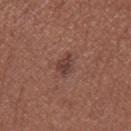Q: Was this lesion biopsied?
A: no biopsy performed (imaged during a skin exam)
Q: Illumination type?
A: white-light illumination
Q: Patient demographics?
A: female, aged around 55
Q: What is the lesion's diameter?
A: ≈2.5 mm
Q: What is the imaging modality?
A: ~15 mm crop, total-body skin-cancer survey
Q: Where on the body is the lesion?
A: the leg